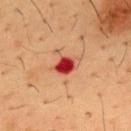Notes:
– notes: total-body-photography surveillance lesion; no biopsy